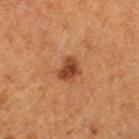notes: catalogued during a skin exam; not biopsied
TBP lesion metrics: a footprint of about 5.5 mm², an eccentricity of roughly 0.55, and two-axis asymmetry of about 0.35; a border-irregularity index near 3/10, a color-variation rating of about 3/10, and peripheral color asymmetry of about 1
lesion size: ~3 mm (longest diameter)
lighting: cross-polarized illumination
anatomic site: the left thigh
patient: female, in their 50s
imaging modality: 15 mm crop, total-body photography